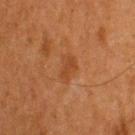<tbp_lesion>
<biopsy_status>not biopsied; imaged during a skin examination</biopsy_status>
<image>
  <source>total-body photography crop</source>
  <field_of_view_mm>15</field_of_view_mm>
</image>
<lesion_size>
  <long_diameter_mm_approx>3.0</long_diameter_mm_approx>
</lesion_size>
<patient>
  <sex>male</sex>
  <age_approx>65</age_approx>
</patient>
<lighting>cross-polarized</lighting>
<site>upper back</site>
</tbp_lesion>